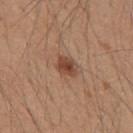Findings:
* notes — no biopsy performed (imaged during a skin exam)
* TBP lesion metrics — a lesion color around L≈45 a*≈21 b*≈30 in CIELAB, a lesion–skin lightness drop of about 11, and a normalized border contrast of about 8.5; a classifier nevus-likeness of about 90/100
* anatomic site — the mid back
* acquisition — total-body-photography crop, ~15 mm field of view
* subject — male, aged approximately 20
* tile lighting — white-light
* lesion size — about 3 mm